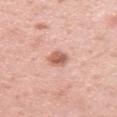biopsy status: imaged on a skin check; not biopsied | imaging modality: ~15 mm tile from a whole-body skin photo | patient: female, about 35 years old | image-analysis metrics: a lesion area of about 4.5 mm², a shape eccentricity near 0.7, and a shape-asymmetry score of about 0.25 (0 = symmetric); about 13 CIELAB-L* units darker than the surrounding skin and a normalized lesion–skin contrast near 8; a classifier nevus-likeness of about 90/100 and a detector confidence of about 100 out of 100 that the crop contains a lesion | lighting: white-light | anatomic site: the right upper arm | size: ≈2.5 mm.